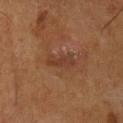Captured during whole-body skin photography for melanoma surveillance; the lesion was not biopsied. Automated tile analysis of the lesion measured an area of roughly 5 mm² and an outline eccentricity of about 0.9 (0 = round, 1 = elongated). And it measured a border-irregularity index near 4.5/10, a within-lesion color-variation index near 1.5/10, and a peripheral color-asymmetry measure near 0.5. And it measured a classifier nevus-likeness of about 0/100 and a lesion-detection confidence of about 100/100. A male patient approximately 55 years of age. A region of skin cropped from a whole-body photographic capture, roughly 15 mm wide. From the front of the torso.